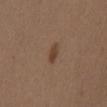Captured during whole-body skin photography for melanoma surveillance; the lesion was not biopsied. Automated image analysis of the tile measured a lesion color around L≈42 a*≈17 b*≈28 in CIELAB, a lesion–skin lightness drop of about 8, and a lesion-to-skin contrast of about 7 (normalized; higher = more distinct). And it measured a border-irregularity rating of about 2/10, a within-lesion color-variation index near 2/10, and peripheral color asymmetry of about 0.5. Cropped from a total-body skin-imaging series; the visible field is about 15 mm. On the mid back. The patient is a female roughly 40 years of age. About 3 mm across. This is a white-light tile.